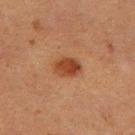- patient: female, approximately 50 years of age
- imaging modality: total-body-photography crop, ~15 mm field of view
- automated lesion analysis: a border-irregularity index near 2/10, a within-lesion color-variation index near 3.5/10, and a peripheral color-asymmetry measure near 1.5; a detector confidence of about 100 out of 100 that the crop contains a lesion
- site: the left thigh
- diameter: ≈3.5 mm